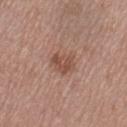{"biopsy_status": "not biopsied; imaged during a skin examination", "lesion_size": {"long_diameter_mm_approx": 3.0}, "lighting": "white-light", "site": "left thigh", "image": {"source": "total-body photography crop", "field_of_view_mm": 15}, "patient": {"sex": "female", "age_approx": 55}, "automated_metrics": {"area_mm2_approx": 6.0, "shape_asymmetry": 0.25, "cielab_L": 50, "cielab_a": 21, "cielab_b": 27, "vs_skin_contrast_norm": 7.0, "nevus_likeness_0_100": 55, "lesion_detection_confidence_0_100": 100}}